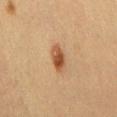biopsy status: catalogued during a skin exam; not biopsied | location: the back | lesion diameter: ~3.5 mm (longest diameter) | patient: female, aged 38 to 42 | acquisition: total-body-photography crop, ~15 mm field of view | illumination: cross-polarized illumination.